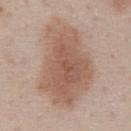The lesion was photographed on a routine skin check and not biopsied; there is no pathology result. Imaged with white-light lighting. Automated tile analysis of the lesion measured a lesion color around L≈57 a*≈19 b*≈27 in CIELAB, a lesion–skin lightness drop of about 11, and a lesion-to-skin contrast of about 7.5 (normalized; higher = more distinct). A lesion tile, about 15 mm wide, cut from a 3D total-body photograph. A male patient aged approximately 60. The lesion is on the abdomen.